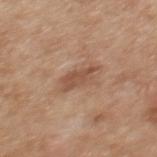{
  "lesion_size": {
    "long_diameter_mm_approx": 4.0
  },
  "patient": {
    "sex": "female",
    "age_approx": 40
  },
  "site": "mid back",
  "image": {
    "source": "total-body photography crop",
    "field_of_view_mm": 15
  },
  "lighting": "white-light"
}